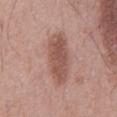The lesion was tiled from a total-body skin photograph and was not biopsied. A 15 mm close-up extracted from a 3D total-body photography capture. A male subject, about 55 years old. On the mid back. The tile uses white-light illumination.